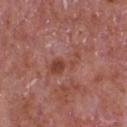Assessment:
Imaged during a routine full-body skin examination; the lesion was not biopsied and no histopathology is available.
Acquisition and patient details:
Captured under white-light illumination. A male subject, aged 63–67. A close-up tile cropped from a whole-body skin photograph, about 15 mm across. The recorded lesion diameter is about 5 mm. From the chest.